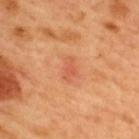• follow-up: total-body-photography surveillance lesion; no biopsy
• patient: male, about 50 years old
• automated metrics: a lesion area of about 4 mm², an outline eccentricity of about 0.8 (0 = round, 1 = elongated), and a shape-asymmetry score of about 0.35 (0 = symmetric); a lesion color around L≈58 a*≈30 b*≈38 in CIELAB, roughly 7 lightness units darker than nearby skin, and a lesion-to-skin contrast of about 4.5 (normalized; higher = more distinct); a nevus-likeness score of about 0/100 and lesion-presence confidence of about 100/100
• lesion size: about 2.5 mm
• tile lighting: cross-polarized
• imaging modality: 15 mm crop, total-body photography
• anatomic site: the upper back